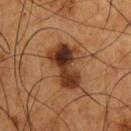follow-up: imaged on a skin check; not biopsied | image: ~15 mm tile from a whole-body skin photo | size: about 6 mm | patient: male, in their mid-50s | illumination: cross-polarized illumination | body site: the front of the torso | automated metrics: a lesion color around L≈33 a*≈20 b*≈30 in CIELAB, a lesion–skin lightness drop of about 14, and a lesion-to-skin contrast of about 12 (normalized; higher = more distinct); border irregularity of about 3.5 on a 0–10 scale, a color-variation rating of about 10/10, and radial color variation of about 3.5.